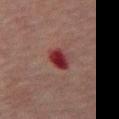Background:
A male subject roughly 60 years of age. The lesion's longest dimension is about 3 mm. A 15 mm close-up tile from a total-body photography series done for melanoma screening. From the abdomen. Imaged with cross-polarized lighting.